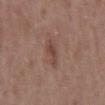This lesion was catalogued during total-body skin photography and was not selected for biopsy. A male patient, aged 48 to 52. The tile uses white-light illumination. From the mid back. The lesion-visualizer software estimated a footprint of about 4 mm², an eccentricity of roughly 0.9, and a shape-asymmetry score of about 0.35 (0 = symmetric). This image is a 15 mm lesion crop taken from a total-body photograph. The lesion's longest dimension is about 3.5 mm.